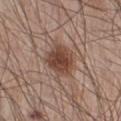Impression: The lesion was tiled from a total-body skin photograph and was not biopsied. Context: On the right lower leg. A male patient aged 58 to 62. The lesion-visualizer software estimated a lesion area of about 10 mm², an eccentricity of roughly 0.5, and a symmetry-axis asymmetry near 0.25. The analysis additionally found a peripheral color-asymmetry measure near 1. And it measured a detector confidence of about 100 out of 100 that the crop contains a lesion. A 15 mm close-up tile from a total-body photography series done for melanoma screening.